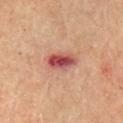Notes:
- follow-up — imaged on a skin check; not biopsied
- patient — male, about 65 years old
- location — the chest
- image — ~15 mm tile from a whole-body skin photo
- TBP lesion metrics — a shape-asymmetry score of about 0.2 (0 = symmetric); a border-irregularity index near 2/10, a color-variation rating of about 8.5/10, and peripheral color asymmetry of about 3; a nevus-likeness score of about 0/100
- size — ≈4 mm